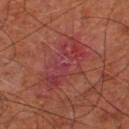Captured during whole-body skin photography for melanoma surveillance; the lesion was not biopsied.
This is a cross-polarized tile.
Automated tile analysis of the lesion measured an area of roughly 19 mm², an eccentricity of roughly 0.9, and a symmetry-axis asymmetry near 0.25. The software also gave an average lesion color of about L≈37 a*≈30 b*≈22 (CIELAB) and a normalized border contrast of about 6.5. The software also gave border irregularity of about 4.5 on a 0–10 scale, a color-variation rating of about 6/10, and radial color variation of about 2. The analysis additionally found a classifier nevus-likeness of about 0/100 and a lesion-detection confidence of about 95/100.
A 15 mm crop from a total-body photograph taken for skin-cancer surveillance.
A male patient aged 63 to 67.
Approximately 7.5 mm at its widest.
From the left lower leg.